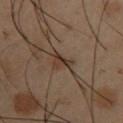biopsy_status: not biopsied; imaged during a skin examination
patient:
  sex: male
  age_approx: 40
image:
  source: total-body photography crop
  field_of_view_mm: 15
site: upper back